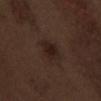Clinical impression:
The lesion was tiled from a total-body skin photograph and was not biopsied.
Acquisition and patient details:
The lesion-visualizer software estimated a footprint of about 5.5 mm². It also reported a mean CIELAB color near L≈20 a*≈15 b*≈18, a lesion–skin lightness drop of about 6, and a normalized border contrast of about 8. It also reported an automated nevus-likeness rating near 70 out of 100. This is a white-light tile. The subject is a male approximately 70 years of age. Cropped from a whole-body photographic skin survey; the tile spans about 15 mm. From the abdomen.